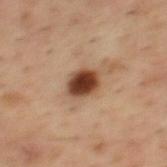biopsy status: no biopsy performed (imaged during a skin exam)
lighting: cross-polarized illumination
TBP lesion metrics: a lesion area of about 8 mm², a shape eccentricity near 0.55, and a shape-asymmetry score of about 0.15 (0 = symmetric); a nevus-likeness score of about 100/100
subject: male, about 55 years old
image: total-body-photography crop, ~15 mm field of view
lesion size: about 3.5 mm
body site: the mid back